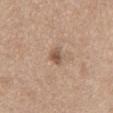Impression: Imaged during a routine full-body skin examination; the lesion was not biopsied and no histopathology is available. Acquisition and patient details: An algorithmic analysis of the crop reported a lesion area of about 3 mm², a shape eccentricity near 0.85, and two-axis asymmetry of about 0.3. It also reported border irregularity of about 3 on a 0–10 scale. It also reported an automated nevus-likeness rating near 50 out of 100 and a detector confidence of about 100 out of 100 that the crop contains a lesion. The tile uses white-light illumination. The lesion is located on the chest. A roughly 15 mm field-of-view crop from a total-body skin photograph. A female subject approximately 65 years of age.